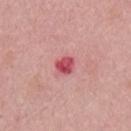• workup: no biopsy performed (imaged during a skin exam)
• patient: male, aged around 50
• image-analysis metrics: an eccentricity of roughly 0.5 and a symmetry-axis asymmetry near 0.15; a border-irregularity index near 1.5/10, a within-lesion color-variation index near 4.5/10, and a peripheral color-asymmetry measure near 1.5
• acquisition: ~15 mm crop, total-body skin-cancer survey
• body site: the chest
• illumination: white-light